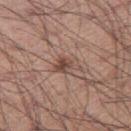{"automated_metrics": {"area_mm2_approx": 4.0, "eccentricity": 0.75, "cielab_L": 47, "cielab_a": 19, "cielab_b": 24, "vs_skin_darker_L": 10.0, "vs_skin_contrast_norm": 8.0}, "site": "right thigh", "lighting": "white-light", "image": {"source": "total-body photography crop", "field_of_view_mm": 15}, "patient": {"sex": "male", "age_approx": 55}}